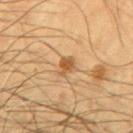Impression: Captured during whole-body skin photography for melanoma surveillance; the lesion was not biopsied. Context: A 15 mm close-up tile from a total-body photography series done for melanoma screening. The lesion is located on the chest. Captured under cross-polarized illumination. About 2.5 mm across. A male subject, roughly 55 years of age.